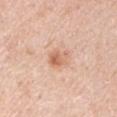Findings:
– biopsy status · total-body-photography surveillance lesion; no biopsy
– subject · male, aged 48 to 52
– automated lesion analysis · an area of roughly 4.5 mm² and a shape-asymmetry score of about 0.25 (0 = symmetric)
– lesion size · ≈3 mm
– site · the right upper arm
– image · ~15 mm crop, total-body skin-cancer survey